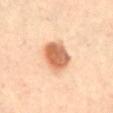This lesion was catalogued during total-body skin photography and was not selected for biopsy. The recorded lesion diameter is about 4 mm. A female patient, in their mid- to late 60s. A close-up tile cropped from a whole-body skin photograph, about 15 mm across. Located on the abdomen.